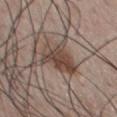<case>
  <biopsy_status>not biopsied; imaged during a skin examination</biopsy_status>
  <site>chest</site>
  <patient>
    <sex>male</sex>
    <age_approx>55</age_approx>
  </patient>
  <image>
    <source>total-body photography crop</source>
    <field_of_view_mm>15</field_of_view_mm>
  </image>
</case>